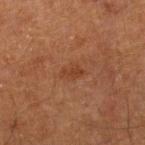{
  "biopsy_status": "not biopsied; imaged during a skin examination",
  "patient": {
    "sex": "male",
    "age_approx": 65
  },
  "automated_metrics": {
    "area_mm2_approx": 3.5,
    "eccentricity": 0.8,
    "shape_asymmetry": 0.3,
    "cielab_L": 32,
    "cielab_a": 20,
    "cielab_b": 28,
    "vs_skin_darker_L": 5.0,
    "vs_skin_contrast_norm": 5.5,
    "nevus_likeness_0_100": 0,
    "lesion_detection_confidence_0_100": 100
  },
  "site": "left lower leg",
  "lesion_size": {
    "long_diameter_mm_approx": 3.0
  },
  "image": {
    "source": "total-body photography crop",
    "field_of_view_mm": 15
  }
}